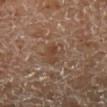{
  "biopsy_status": "not biopsied; imaged during a skin examination",
  "image": {
    "source": "total-body photography crop",
    "field_of_view_mm": 15
  },
  "patient": {
    "sex": "male",
    "age_approx": 55
  },
  "lesion_size": {
    "long_diameter_mm_approx": 3.5
  },
  "lighting": "cross-polarized",
  "automated_metrics": {
    "peripheral_color_asymmetry": 1.5
  },
  "site": "left lower leg"
}